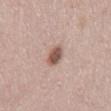Q: Is there a histopathology result?
A: no biopsy performed (imaged during a skin exam)
Q: What is the anatomic site?
A: the mid back
Q: Illumination type?
A: white-light
Q: How was this image acquired?
A: ~15 mm tile from a whole-body skin photo
Q: What are the patient's age and sex?
A: female, approximately 30 years of age